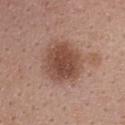{
  "biopsy_status": "not biopsied; imaged during a skin examination",
  "patient": {
    "sex": "female",
    "age_approx": 40
  },
  "automated_metrics": {
    "cielab_L": 48,
    "cielab_a": 21,
    "cielab_b": 26,
    "vs_skin_darker_L": 12.0,
    "vs_skin_contrast_norm": 8.5,
    "nevus_likeness_0_100": 75,
    "lesion_detection_confidence_0_100": 100
  },
  "site": "upper back",
  "lighting": "white-light",
  "image": {
    "source": "total-body photography crop",
    "field_of_view_mm": 15
  },
  "lesion_size": {
    "long_diameter_mm_approx": 5.0
  }
}